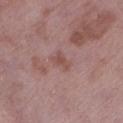Notes:
* follow-up · catalogued during a skin exam; not biopsied
* size · ~2.5 mm (longest diameter)
* patient · female, aged 68 to 72
* tile lighting · white-light
* automated lesion analysis · a lesion color around L≈50 a*≈21 b*≈22 in CIELAB, about 7 CIELAB-L* units darker than the surrounding skin, and a normalized border contrast of about 6; a border-irregularity index near 3.5/10, a color-variation rating of about 0.5/10, and radial color variation of about 0; a classifier nevus-likeness of about 0/100 and lesion-presence confidence of about 100/100
* imaging modality · total-body-photography crop, ~15 mm field of view
* site · the left lower leg